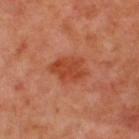biopsy status = no biopsy performed (imaged during a skin exam); patient = male, aged around 50; image = 15 mm crop, total-body photography; anatomic site = the upper back.